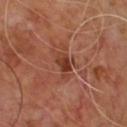- biopsy status — no biopsy performed (imaged during a skin exam)
- subject — male, aged around 65
- acquisition — ~15 mm crop, total-body skin-cancer survey
- diameter — ~3.5 mm (longest diameter)
- illumination — cross-polarized